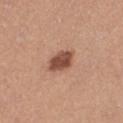Q: Was this lesion biopsied?
A: total-body-photography surveillance lesion; no biopsy
Q: Automated lesion metrics?
A: a lesion area of about 6.5 mm², an outline eccentricity of about 0.75 (0 = round, 1 = elongated), and a symmetry-axis asymmetry near 0.2; a mean CIELAB color near L≈49 a*≈23 b*≈29 and a lesion–skin lightness drop of about 15; a border-irregularity rating of about 2/10 and internal color variation of about 2.5 on a 0–10 scale
Q: Where on the body is the lesion?
A: the leg
Q: How was the tile lit?
A: white-light
Q: Lesion size?
A: ~3 mm (longest diameter)
Q: What kind of image is this?
A: ~15 mm tile from a whole-body skin photo
Q: What are the patient's age and sex?
A: female, about 35 years old